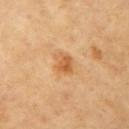No biopsy was performed on this lesion — it was imaged during a full skin examination and was not determined to be concerning. Approximately 3 mm at its widest. Cropped from a total-body skin-imaging series; the visible field is about 15 mm. A female subject aged 63–67. An algorithmic analysis of the crop reported an outline eccentricity of about 0.7 (0 = round, 1 = elongated) and two-axis asymmetry of about 0.25. It also reported border irregularity of about 2.5 on a 0–10 scale, a color-variation rating of about 4.5/10, and radial color variation of about 2. Captured under cross-polarized illumination. Located on the left upper arm.